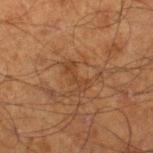Part of a total-body skin-imaging series; this lesion was reviewed on a skin check and was not flagged for biopsy.
This image is a 15 mm lesion crop taken from a total-body photograph.
The lesion is on the right lower leg.
The recorded lesion diameter is about 3.5 mm.
Imaged with cross-polarized lighting.
A male patient about 70 years old.
The total-body-photography lesion software estimated a lesion–skin lightness drop of about 5 and a normalized lesion–skin contrast near 5.